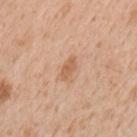Clinical impression:
The lesion was photographed on a routine skin check and not biopsied; there is no pathology result.
Image and clinical context:
Located on the back. This image is a 15 mm lesion crop taken from a total-body photograph. Automated tile analysis of the lesion measured a symmetry-axis asymmetry near 0.25. It also reported a color-variation rating of about 1/10 and a peripheral color-asymmetry measure near 0.5. Imaged with white-light lighting. Longest diameter approximately 2.5 mm. A male patient, in their mid-60s.